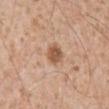No biopsy was performed on this lesion — it was imaged during a full skin examination and was not determined to be concerning. A close-up tile cropped from a whole-body skin photograph, about 15 mm across. The lesion is located on the abdomen. The subject is a male about 65 years old. About 2.5 mm across. Imaged with white-light lighting.